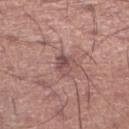| key | value |
|---|---|
| biopsy status | total-body-photography surveillance lesion; no biopsy |
| subject | male, aged 43–47 |
| automated lesion analysis | an average lesion color of about L≈49 a*≈21 b*≈21 (CIELAB), roughly 9 lightness units darker than nearby skin, and a lesion-to-skin contrast of about 6.5 (normalized; higher = more distinct); a border-irregularity index near 2.5/10, a within-lesion color-variation index near 5/10, and peripheral color asymmetry of about 2; a classifier nevus-likeness of about 0/100 and a detector confidence of about 85 out of 100 that the crop contains a lesion |
| lighting | white-light |
| lesion diameter | ~2.5 mm (longest diameter) |
| anatomic site | the leg |
| acquisition | ~15 mm tile from a whole-body skin photo |